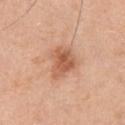Impression: Imaged during a routine full-body skin examination; the lesion was not biopsied and no histopathology is available. Image and clinical context: This is a white-light tile. A 15 mm close-up extracted from a 3D total-body photography capture. A male patient approximately 50 years of age. The total-body-photography lesion software estimated a mean CIELAB color near L≈58 a*≈24 b*≈34, roughly 12 lightness units darker than nearby skin, and a normalized border contrast of about 7.5. It also reported a border-irregularity index near 3.5/10, internal color variation of about 5 on a 0–10 scale, and a peripheral color-asymmetry measure near 1.5. From the chest.